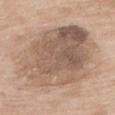Captured during whole-body skin photography for melanoma surveillance; the lesion was not biopsied.
This image is a 15 mm lesion crop taken from a total-body photograph.
A female subject aged approximately 75.
The lesion is located on the upper back.